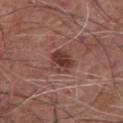Part of a total-body skin-imaging series; this lesion was reviewed on a skin check and was not flagged for biopsy. A male subject aged around 65. On the chest. Approximately 3 mm at its widest. Automated image analysis of the tile measured a footprint of about 6.5 mm², an eccentricity of roughly 0.5, and a symmetry-axis asymmetry near 0.2. And it measured a within-lesion color-variation index near 4.5/10. Cropped from a total-body skin-imaging series; the visible field is about 15 mm.